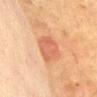<tbp_lesion>
<biopsy_status>not biopsied; imaged during a skin examination</biopsy_status>
<lesion_size>
  <long_diameter_mm_approx>4.5</long_diameter_mm_approx>
</lesion_size>
<site>front of the torso</site>
<patient>
  <sex>female</sex>
  <age_approx>50</age_approx>
</patient>
<image>
  <source>total-body photography crop</source>
  <field_of_view_mm>15</field_of_view_mm>
</image>
<lighting>cross-polarized</lighting>
</tbp_lesion>